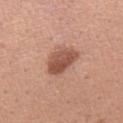• workup — imaged on a skin check; not biopsied
• subject — female, aged 28–32
• image-analysis metrics — a lesion area of about 9.5 mm², an outline eccentricity of about 0.75 (0 = round, 1 = elongated), and a symmetry-axis asymmetry near 0.25; an average lesion color of about L≈52 a*≈24 b*≈29 (CIELAB) and roughly 13 lightness units darker than nearby skin; border irregularity of about 2 on a 0–10 scale, a color-variation rating of about 4.5/10, and a peripheral color-asymmetry measure near 1.5; a classifier nevus-likeness of about 55/100
• imaging modality — ~15 mm tile from a whole-body skin photo
• site — the left forearm
• lighting — white-light
• lesion size — about 4.5 mm